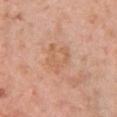Impression: No biopsy was performed on this lesion — it was imaged during a full skin examination and was not determined to be concerning. Image and clinical context: The lesion is on the chest. The lesion's longest dimension is about 4 mm. Imaged with white-light lighting. A close-up tile cropped from a whole-body skin photograph, about 15 mm across. A female subject aged 38–42.